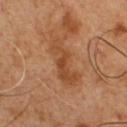This lesion was catalogued during total-body skin photography and was not selected for biopsy. About 6.5 mm across. A male subject aged 48–52. The lesion is located on the chest. This is a cross-polarized tile. A lesion tile, about 15 mm wide, cut from a 3D total-body photograph. Automated tile analysis of the lesion measured an area of roughly 11 mm², a shape eccentricity near 0.95, and a shape-asymmetry score of about 0.4 (0 = symmetric). It also reported an average lesion color of about L≈47 a*≈23 b*≈37 (CIELAB) and a normalized border contrast of about 7. And it measured a classifier nevus-likeness of about 0/100 and a detector confidence of about 100 out of 100 that the crop contains a lesion.